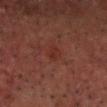follow-up: imaged on a skin check; not biopsied | subject: male, in their 50s | body site: the head or neck | acquisition: total-body-photography crop, ~15 mm field of view.